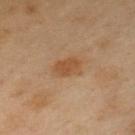biopsy_status: not biopsied; imaged during a skin examination
image:
  source: total-body photography crop
  field_of_view_mm: 15
site: chest
automated_metrics:
  border_irregularity_0_10: 2.0
  peripheral_color_asymmetry: 0.5
  nevus_likeness_0_100: 70
patient:
  sex: male
  age_approx: 55
lesion_size:
  long_diameter_mm_approx: 3.5